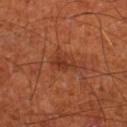The lesion was photographed on a routine skin check and not biopsied; there is no pathology result. The total-body-photography lesion software estimated a lesion area of about 3.5 mm², an outline eccentricity of about 0.8 (0 = round, 1 = elongated), and a symmetry-axis asymmetry near 0.4. And it measured an automated nevus-likeness rating near 25 out of 100 and lesion-presence confidence of about 100/100. A male patient in their mid- to late 60s. Longest diameter approximately 3 mm. A lesion tile, about 15 mm wide, cut from a 3D total-body photograph. On the left lower leg.